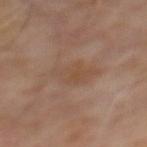Recorded during total-body skin imaging; not selected for excision or biopsy.
From the mid back.
Automated tile analysis of the lesion measured border irregularity of about 4.5 on a 0–10 scale, a within-lesion color-variation index near 3/10, and radial color variation of about 1. The software also gave an automated nevus-likeness rating near 0 out of 100.
Longest diameter approximately 5 mm.
A male subject, about 65 years old.
A close-up tile cropped from a whole-body skin photograph, about 15 mm across.